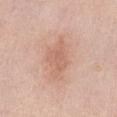Recorded during total-body skin imaging; not selected for excision or biopsy.
A female patient about 50 years old.
Located on the abdomen.
An algorithmic analysis of the crop reported a lesion area of about 7.5 mm² and a shape eccentricity near 0.8. The analysis additionally found a mean CIELAB color near L≈63 a*≈22 b*≈29, roughly 8 lightness units darker than nearby skin, and a normalized lesion–skin contrast near 5.5.
Approximately 4.5 mm at its widest.
A lesion tile, about 15 mm wide, cut from a 3D total-body photograph.